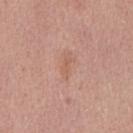Captured during whole-body skin photography for melanoma surveillance; the lesion was not biopsied. Approximately 2.5 mm at its widest. On the front of the torso. The tile uses white-light illumination. A 15 mm close-up extracted from a 3D total-body photography capture. The subject is a male in their mid-20s. Automated image analysis of the tile measured a lesion color around L≈59 a*≈22 b*≈29 in CIELAB, about 6 CIELAB-L* units darker than the surrounding skin, and a normalized border contrast of about 4.5.